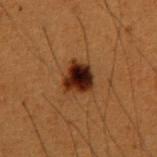Case summary:
- follow-up · total-body-photography surveillance lesion; no biopsy
- location · the left upper arm
- patient · male, approximately 50 years of age
- image · 15 mm crop, total-body photography